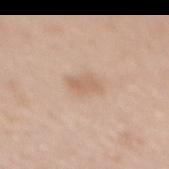Acquisition and patient details: The total-body-photography lesion software estimated a footprint of about 4 mm², an outline eccentricity of about 0.85 (0 = round, 1 = elongated), and two-axis asymmetry of about 0.3. The software also gave border irregularity of about 3 on a 0–10 scale, a color-variation rating of about 1.5/10, and a peripheral color-asymmetry measure near 0.5. It also reported a classifier nevus-likeness of about 15/100 and a detector confidence of about 100 out of 100 that the crop contains a lesion. Located on the mid back. The subject is a female aged 58 to 62. Captured under white-light illumination. A lesion tile, about 15 mm wide, cut from a 3D total-body photograph.